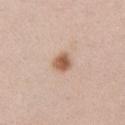<case>
<biopsy_status>not biopsied; imaged during a skin examination</biopsy_status>
<site>left upper arm</site>
<image>
  <source>total-body photography crop</source>
  <field_of_view_mm>15</field_of_view_mm>
</image>
<lesion_size>
  <long_diameter_mm_approx>2.5</long_diameter_mm_approx>
</lesion_size>
<patient>
  <sex>female</sex>
  <age_approx>40</age_approx>
</patient>
</case>